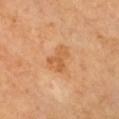* body site · the front of the torso
* subject · female, aged approximately 70
* TBP lesion metrics · a mean CIELAB color near L≈61 a*≈25 b*≈43 and a lesion–skin lightness drop of about 8
* tile lighting · cross-polarized illumination
* diameter · about 3.5 mm
* image source · total-body-photography crop, ~15 mm field of view